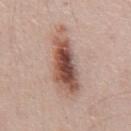follow-up = catalogued during a skin exam; not biopsied | subject = male, aged approximately 55 | anatomic site = the front of the torso | lighting = white-light illumination | imaging modality = ~15 mm crop, total-body skin-cancer survey | size = ~8 mm (longest diameter).